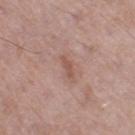Assessment: Recorded during total-body skin imaging; not selected for excision or biopsy. Background: A male subject, aged 53 to 57. The lesion is located on the right thigh. A lesion tile, about 15 mm wide, cut from a 3D total-body photograph.